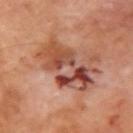biopsy status: total-body-photography surveillance lesion; no biopsy